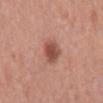The lesion was photographed on a routine skin check and not biopsied; there is no pathology result.
This is a white-light tile.
A male subject, aged approximately 70.
The lesion's longest dimension is about 3.5 mm.
This image is a 15 mm lesion crop taken from a total-body photograph.
The lesion-visualizer software estimated border irregularity of about 2 on a 0–10 scale, a within-lesion color-variation index near 2.5/10, and peripheral color asymmetry of about 0.5. It also reported a detector confidence of about 100 out of 100 that the crop contains a lesion.
Located on the mid back.